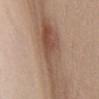biopsy_status: not biopsied; imaged during a skin examination
lesion_size:
  long_diameter_mm_approx: 9.0
site: chest
lighting: white-light
image:
  source: total-body photography crop
  field_of_view_mm: 15
patient:
  sex: female
  age_approx: 40